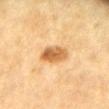No biopsy was performed on this lesion — it was imaged during a full skin examination and was not determined to be concerning.
The lesion is on the chest.
A female subject, aged approximately 55.
A 15 mm crop from a total-body photograph taken for skin-cancer surveillance.
Longest diameter approximately 3.5 mm.
Captured under cross-polarized illumination.